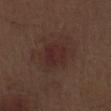biopsy_status: not biopsied; imaged during a skin examination
lighting: white-light
lesion_size:
  long_diameter_mm_approx: 3.0
image:
  source: total-body photography crop
  field_of_view_mm: 15
patient:
  sex: male
  age_approx: 70
site: right thigh